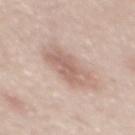| field | value |
|---|---|
| workup | imaged on a skin check; not biopsied |
| tile lighting | white-light |
| size | about 5.5 mm |
| acquisition | total-body-photography crop, ~15 mm field of view |
| TBP lesion metrics | a lesion area of about 13 mm²; border irregularity of about 3 on a 0–10 scale; a nevus-likeness score of about 15/100 and a detector confidence of about 100 out of 100 that the crop contains a lesion |
| location | the mid back |
| subject | male, roughly 45 years of age |